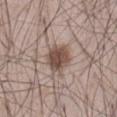This lesion was catalogued during total-body skin photography and was not selected for biopsy.
A roughly 15 mm field-of-view crop from a total-body skin photograph.
Imaged with white-light lighting.
Measured at roughly 4 mm in maximum diameter.
The lesion is located on the abdomen.
A male subject, in their 50s.